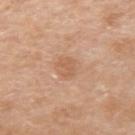biopsy_status: not biopsied; imaged during a skin examination
image:
  source: total-body photography crop
  field_of_view_mm: 15
lesion_size:
  long_diameter_mm_approx: 2.5
patient:
  sex: male
  age_approx: 60
automated_metrics:
  area_mm2_approx: 4.0
  eccentricity: 0.75
  shape_asymmetry: 0.3
  vs_skin_darker_L: 7.0
  vs_skin_contrast_norm: 5.0
  border_irregularity_0_10: 3.5
  color_variation_0_10: 1.0
  peripheral_color_asymmetry: 0.5
  nevus_likeness_0_100: 0
  lesion_detection_confidence_0_100: 100
site: left upper arm